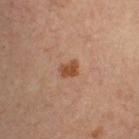biopsy status = catalogued during a skin exam; not biopsied | TBP lesion metrics = a footprint of about 3.5 mm², a shape eccentricity near 0.6, and a symmetry-axis asymmetry near 0.3; a border-irregularity index near 2.5/10 and a within-lesion color-variation index near 3/10 | diameter = about 2.5 mm | body site = the left upper arm | tile lighting = cross-polarized illumination | patient = male, aged 63–67 | imaging modality = 15 mm crop, total-body photography.